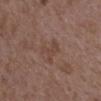anatomic site: the upper back
tile lighting: white-light illumination
lesion size: ≈3.5 mm
imaging modality: ~15 mm crop, total-body skin-cancer survey
subject: female, aged approximately 35